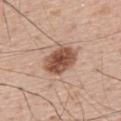Captured during whole-body skin photography for melanoma surveillance; the lesion was not biopsied. The lesion's longest dimension is about 4.5 mm. The lesion-visualizer software estimated a mean CIELAB color near L≈51 a*≈22 b*≈29 and about 16 CIELAB-L* units darker than the surrounding skin. This is a white-light tile. The lesion is on the back. A 15 mm close-up extracted from a 3D total-body photography capture. A male subject, roughly 65 years of age.